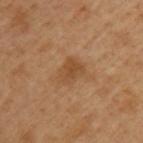notes = total-body-photography surveillance lesion; no biopsy
location = the arm
patient = male, aged 48 to 52
imaging modality = ~15 mm crop, total-body skin-cancer survey
diameter = ≈3.5 mm